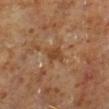Findings:
- notes · imaged on a skin check; not biopsied
- automated lesion analysis · a lesion color around L≈40 a*≈19 b*≈32 in CIELAB and a lesion-to-skin contrast of about 7 (normalized; higher = more distinct); a nevus-likeness score of about 0/100 and a detector confidence of about 90 out of 100 that the crop contains a lesion
- image source · total-body-photography crop, ~15 mm field of view
- patient · male, aged 58–62
- location · the left lower leg
- diameter · about 3 mm
- tile lighting · cross-polarized